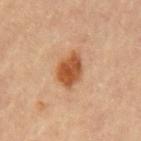- image — 15 mm crop, total-body photography
- lesion size — ≈4 mm
- patient — female, in their mid- to late 60s
- body site — the left upper arm
- lighting — cross-polarized illumination
- TBP lesion metrics — a lesion area of about 8.5 mm² and two-axis asymmetry of about 0.2; a border-irregularity index near 2/10, a within-lesion color-variation index near 3.5/10, and a peripheral color-asymmetry measure near 1